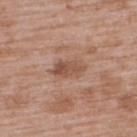Clinical impression:
This lesion was catalogued during total-body skin photography and was not selected for biopsy.
Context:
The lesion is located on the upper back. A 15 mm close-up tile from a total-body photography series done for melanoma screening. Imaged with white-light lighting. The patient is a male about 50 years old. The recorded lesion diameter is about 4 mm.